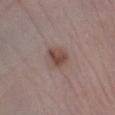| field | value |
|---|---|
| biopsy status | total-body-photography surveillance lesion; no biopsy |
| anatomic site | the left lower leg |
| image source | ~15 mm crop, total-body skin-cancer survey |
| lighting | white-light illumination |
| subject | female, approximately 65 years of age |
| diameter | ~3 mm (longest diameter) |